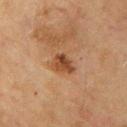Impression: The lesion was photographed on a routine skin check and not biopsied; there is no pathology result. Background: On the upper back. This is a cross-polarized tile. A 15 mm close-up tile from a total-body photography series done for melanoma screening. Approximately 2.5 mm at its widest. A male subject, aged approximately 75.